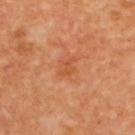Clinical impression: Recorded during total-body skin imaging; not selected for excision or biopsy. Image and clinical context: The tile uses cross-polarized illumination. Cropped from a total-body skin-imaging series; the visible field is about 15 mm. A male patient, aged 63 to 67. Longest diameter approximately 2.5 mm. On the upper back.